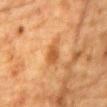Assessment: This lesion was catalogued during total-body skin photography and was not selected for biopsy. Clinical summary: From the chest. A 15 mm crop from a total-body photograph taken for skin-cancer surveillance. A male subject, roughly 85 years of age or older. The recorded lesion diameter is about 3.5 mm.